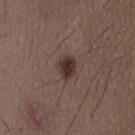Clinical impression: Recorded during total-body skin imaging; not selected for excision or biopsy. Acquisition and patient details: This image is a 15 mm lesion crop taken from a total-body photograph. A male subject in their 50s. This is a white-light tile. Measured at roughly 3 mm in maximum diameter. The lesion is located on the lower back.